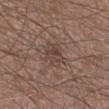Clinical impression: Imaged during a routine full-body skin examination; the lesion was not biopsied and no histopathology is available. Context: An algorithmic analysis of the crop reported a footprint of about 6 mm², an outline eccentricity of about 0.6 (0 = round, 1 = elongated), and a shape-asymmetry score of about 0.2 (0 = symmetric). The software also gave a classifier nevus-likeness of about 0/100 and a lesion-detection confidence of about 95/100. A 15 mm close-up extracted from a 3D total-body photography capture. The lesion is located on the leg. Approximately 3 mm at its widest. The tile uses white-light illumination. The subject is a male about 60 years old.